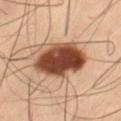The lesion was photographed on a routine skin check and not biopsied; there is no pathology result. Cropped from a whole-body photographic skin survey; the tile spans about 15 mm. From the left thigh. The subject is a male aged 53–57.